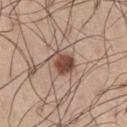Q: Is there a histopathology result?
A: catalogued during a skin exam; not biopsied
Q: Where on the body is the lesion?
A: the leg
Q: What lighting was used for the tile?
A: white-light illumination
Q: Who is the patient?
A: male, in their mid-50s
Q: What is the lesion's diameter?
A: ~3.5 mm (longest diameter)
Q: What kind of image is this?
A: ~15 mm tile from a whole-body skin photo